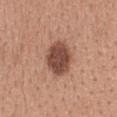Assessment:
Captured during whole-body skin photography for melanoma surveillance; the lesion was not biopsied.
Background:
A lesion tile, about 15 mm wide, cut from a 3D total-body photograph. The recorded lesion diameter is about 4 mm. This is a white-light tile. Located on the upper back. A female patient aged 28 to 32. The lesion-visualizer software estimated border irregularity of about 1 on a 0–10 scale and a color-variation rating of about 3.5/10.